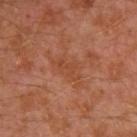<lesion>
  <biopsy_status>not biopsied; imaged during a skin examination</biopsy_status>
  <image>
    <source>total-body photography crop</source>
    <field_of_view_mm>15</field_of_view_mm>
  </image>
  <patient>
    <sex>male</sex>
    <age_approx>30</age_approx>
  </patient>
  <automated_metrics>
    <area_mm2_approx>4.5</area_mm2_approx>
    <eccentricity>0.8</eccentricity>
    <shape_asymmetry>0.4</shape_asymmetry>
    <vs_skin_darker_L>5.0</vs_skin_darker_L>
    <vs_skin_contrast_norm>4.5</vs_skin_contrast_norm>
    <nevus_likeness_0_100>0</nevus_likeness_0_100>
    <lesion_detection_confidence_0_100>100</lesion_detection_confidence_0_100>
  </automated_metrics>
  <lesion_size>
    <long_diameter_mm_approx>3.5</long_diameter_mm_approx>
  </lesion_size>
  <site>left arm</site>
  <lighting>cross-polarized</lighting>
</lesion>